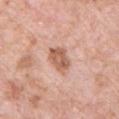Notes:
– workup: imaged on a skin check; not biopsied
– anatomic site: the chest
– subject: male, roughly 50 years of age
– image: total-body-photography crop, ~15 mm field of view
– illumination: white-light